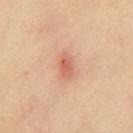Clinical summary: The tile uses cross-polarized illumination. Automated image analysis of the tile measured a footprint of about 4 mm² and two-axis asymmetry of about 0.15. The software also gave a mean CIELAB color near L≈63 a*≈28 b*≈33, a lesion–skin lightness drop of about 11, and a normalized border contrast of about 6.5. The software also gave a nevus-likeness score of about 0/100 and lesion-presence confidence of about 100/100. A 15 mm close-up tile from a total-body photography series done for melanoma screening. The lesion is on the chest.